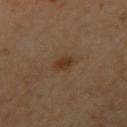Recorded during total-body skin imaging; not selected for excision or biopsy.
Captured under cross-polarized illumination.
On the arm.
The patient is a male aged 63–67.
Automated tile analysis of the lesion measured a lesion area of about 3.5 mm², an outline eccentricity of about 0.7 (0 = round, 1 = elongated), and two-axis asymmetry of about 0.15. The analysis additionally found a mean CIELAB color near L≈35 a*≈17 b*≈29 and a lesion-to-skin contrast of about 7.5 (normalized; higher = more distinct). The software also gave border irregularity of about 1.5 on a 0–10 scale, internal color variation of about 1.5 on a 0–10 scale, and a peripheral color-asymmetry measure near 0.5.
Measured at roughly 2.5 mm in maximum diameter.
A close-up tile cropped from a whole-body skin photograph, about 15 mm across.